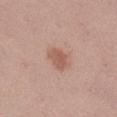Captured during whole-body skin photography for melanoma surveillance; the lesion was not biopsied. Imaged with white-light lighting. A female patient, approximately 35 years of age. A 15 mm close-up extracted from a 3D total-body photography capture. The lesion is on the leg. Automated tile analysis of the lesion measured a color-variation rating of about 2/10 and radial color variation of about 0.5. The software also gave a classifier nevus-likeness of about 90/100 and a detector confidence of about 100 out of 100 that the crop contains a lesion.